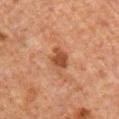notes: no biopsy performed (imaged during a skin exam)
site: the chest
subject: male, aged 58–62
lighting: cross-polarized illumination
size: about 3 mm
image source: ~15 mm tile from a whole-body skin photo